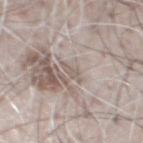No biopsy was performed on this lesion — it was imaged during a full skin examination and was not determined to be concerning. The patient is a male aged 68 to 72. A close-up tile cropped from a whole-body skin photograph, about 15 mm across. The lesion is on the chest.